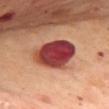Automated image analysis of the tile measured a mean CIELAB color near L≈44 a*≈34 b*≈27, roughly 21 lightness units darker than nearby skin, and a normalized lesion–skin contrast near 14.5. The software also gave a detector confidence of about 100 out of 100 that the crop contains a lesion. Captured under cross-polarized illumination. A female subject in their mid- to late 50s. Cropped from a whole-body photographic skin survey; the tile spans about 15 mm. From the mid back. Measured at roughly 6.5 mm in maximum diameter.